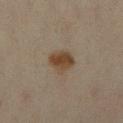follow-up: no biopsy performed (imaged during a skin exam)
lighting: cross-polarized
acquisition: total-body-photography crop, ~15 mm field of view
automated metrics: an outline eccentricity of about 0.65 (0 = round, 1 = elongated); a peripheral color-asymmetry measure near 1
subject: female, aged approximately 45
location: the leg
lesion diameter: ~3 mm (longest diameter)